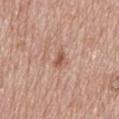Imaged during a routine full-body skin examination; the lesion was not biopsied and no histopathology is available.
A region of skin cropped from a whole-body photographic capture, roughly 15 mm wide.
A male subject, roughly 60 years of age.
On the mid back.